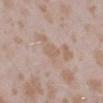Findings:
• notes — catalogued during a skin exam; not biopsied
• patient — female, aged around 25
• lighting — white-light illumination
• site — the leg
• image-analysis metrics — a shape eccentricity near 0.75 and two-axis asymmetry of about 0.2; a border-irregularity index near 2/10 and a peripheral color-asymmetry measure near 0; lesion-presence confidence of about 90/100
• imaging modality — 15 mm crop, total-body photography